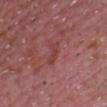Recorded during total-body skin imaging; not selected for excision or biopsy. Captured under white-light illumination. A close-up tile cropped from a whole-body skin photograph, about 15 mm across. A male subject, aged approximately 65. The recorded lesion diameter is about 3 mm. The lesion is located on the chest.